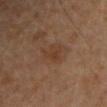Q: Was a biopsy performed?
A: no biopsy performed (imaged during a skin exam)
Q: Where on the body is the lesion?
A: the left upper arm
Q: Automated lesion metrics?
A: an outline eccentricity of about 0.55 (0 = round, 1 = elongated) and a shape-asymmetry score of about 0.4 (0 = symmetric); an average lesion color of about L≈35 a*≈17 b*≈28 (CIELAB), a lesion–skin lightness drop of about 5, and a lesion-to-skin contrast of about 6 (normalized; higher = more distinct); a border-irregularity rating of about 4.5/10 and a color-variation rating of about 1.5/10; a nevus-likeness score of about 5/100
Q: How large is the lesion?
A: ≈3 mm
Q: What are the patient's age and sex?
A: male, about 45 years old
Q: What kind of image is this?
A: total-body-photography crop, ~15 mm field of view
Q: What lighting was used for the tile?
A: cross-polarized illumination